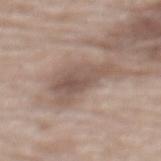The lesion was tiled from a total-body skin photograph and was not biopsied.
Automated tile analysis of the lesion measured a lesion color around L≈54 a*≈15 b*≈24 in CIELAB, about 10 CIELAB-L* units darker than the surrounding skin, and a normalized lesion–skin contrast near 7. And it measured a nevus-likeness score of about 0/100 and a lesion-detection confidence of about 90/100.
Approximately 7 mm at its widest.
Cropped from a total-body skin-imaging series; the visible field is about 15 mm.
This is a white-light tile.
On the mid back.
A female patient, approximately 75 years of age.